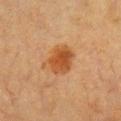{"biopsy_status": "not biopsied; imaged during a skin examination", "site": "chest", "patient": {"sex": "female", "age_approx": 55}, "image": {"source": "total-body photography crop", "field_of_view_mm": 15}}